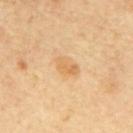The lesion was photographed on a routine skin check and not biopsied; there is no pathology result.
Imaged with cross-polarized lighting.
The recorded lesion diameter is about 3.5 mm.
A male subject, roughly 60 years of age.
The lesion is located on the mid back.
This image is a 15 mm lesion crop taken from a total-body photograph.
The total-body-photography lesion software estimated a footprint of about 5 mm², a shape eccentricity near 0.8, and a shape-asymmetry score of about 0.2 (0 = symmetric). It also reported a lesion color around L≈66 a*≈18 b*≈41 in CIELAB, roughly 8 lightness units darker than nearby skin, and a normalized border contrast of about 5.5. It also reported a nevus-likeness score of about 10/100 and a detector confidence of about 100 out of 100 that the crop contains a lesion.